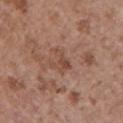Findings:
* follow-up · imaged on a skin check; not biopsied
* anatomic site · the front of the torso
* patient · female, aged 63 to 67
* imaging modality · 15 mm crop, total-body photography
* lighting · white-light illumination
* diameter · ≈3 mm